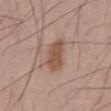<lesion>
  <biopsy_status>not biopsied; imaged during a skin examination</biopsy_status>
  <site>mid back</site>
  <image>
    <source>total-body photography crop</source>
    <field_of_view_mm>15</field_of_view_mm>
  </image>
  <patient>
    <sex>male</sex>
    <age_approx>55</age_approx>
  </patient>
</lesion>